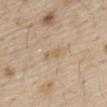Captured during whole-body skin photography for melanoma surveillance; the lesion was not biopsied.
A male patient, roughly 70 years of age.
An algorithmic analysis of the crop reported a lesion area of about 3 mm², an outline eccentricity of about 0.9 (0 = round, 1 = elongated), and a symmetry-axis asymmetry near 0.3. The software also gave a within-lesion color-variation index near 0/10 and peripheral color asymmetry of about 0.
From the abdomen.
A lesion tile, about 15 mm wide, cut from a 3D total-body photograph.
Imaged with white-light lighting.
Measured at roughly 2.5 mm in maximum diameter.